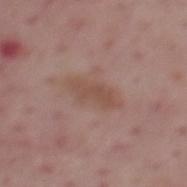{"site": "mid back", "lighting": "white-light", "image": {"source": "total-body photography crop", "field_of_view_mm": 15}, "lesion_size": {"long_diameter_mm_approx": 5.0}, "patient": {"sex": "male", "age_approx": 55}}